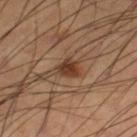Clinical summary:
An algorithmic analysis of the crop reported a border-irregularity index near 2/10, a color-variation rating of about 3/10, and a peripheral color-asymmetry measure near 1. It also reported a classifier nevus-likeness of about 85/100 and a lesion-detection confidence of about 100/100. On the leg. A lesion tile, about 15 mm wide, cut from a 3D total-body photograph. This is a cross-polarized tile. A male subject roughly 65 years of age.